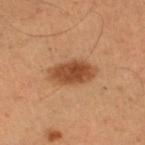image:
  source: total-body photography crop
  field_of_view_mm: 15
lighting: cross-polarized
patient:
  sex: female
  age_approx: 55
lesion_size:
  long_diameter_mm_approx: 5.0
site: leg